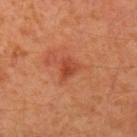  biopsy_status: not biopsied; imaged during a skin examination
  image:
    source: total-body photography crop
    field_of_view_mm: 15
  site: left upper arm
  patient:
    sex: female
    age_approx: 40
  lesion_size:
    long_diameter_mm_approx: 2.5
  automated_metrics:
    area_mm2_approx: 4.0
    eccentricity: 0.6
    shape_asymmetry: 0.4
    cielab_L: 47
    cielab_a: 32
    cielab_b: 37
    vs_skin_darker_L: 9.0
    vs_skin_contrast_norm: 6.5
    border_irregularity_0_10: 3.5
    color_variation_0_10: 2.5
    peripheral_color_asymmetry: 1.0
    nevus_likeness_0_100: 20
    lesion_detection_confidence_0_100: 100